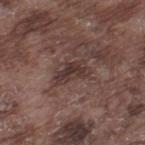biopsy_status: not biopsied; imaged during a skin examination
lesion_size:
  long_diameter_mm_approx: 4.0
patient:
  sex: male
  age_approx: 75
site: left thigh
image:
  source: total-body photography crop
  field_of_view_mm: 15